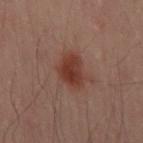Part of a total-body skin-imaging series; this lesion was reviewed on a skin check and was not flagged for biopsy. The subject is a male approximately 40 years of age. Captured under cross-polarized illumination. The recorded lesion diameter is about 4.5 mm. Automated image analysis of the tile measured internal color variation of about 3 on a 0–10 scale and radial color variation of about 1. A region of skin cropped from a whole-body photographic capture, roughly 15 mm wide. The lesion is on the right thigh.